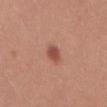The lesion was tiled from a total-body skin photograph and was not biopsied.
A 15 mm close-up extracted from a 3D total-body photography capture.
Automated image analysis of the tile measured a footprint of about 4 mm², an outline eccentricity of about 0.7 (0 = round, 1 = elongated), and a shape-asymmetry score of about 0.2 (0 = symmetric). The software also gave a mean CIELAB color near L≈51 a*≈26 b*≈28 and a normalized lesion–skin contrast near 7.5. The software also gave an automated nevus-likeness rating near 95 out of 100 and a detector confidence of about 100 out of 100 that the crop contains a lesion.
A female subject aged 23–27.
From the abdomen.
This is a white-light tile.